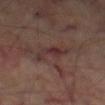Impression: The lesion was tiled from a total-body skin photograph and was not biopsied. Background: From the left thigh. The patient is a male aged 68–72. Captured under cross-polarized illumination. A lesion tile, about 15 mm wide, cut from a 3D total-body photograph. An algorithmic analysis of the crop reported a border-irregularity index near 5/10, a within-lesion color-variation index near 3.5/10, and a peripheral color-asymmetry measure near 1. The software also gave an automated nevus-likeness rating near 0 out of 100.